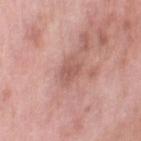Impression: Captured during whole-body skin photography for melanoma surveillance; the lesion was not biopsied. Acquisition and patient details: On the leg. This image is a 15 mm lesion crop taken from a total-body photograph. A female subject about 70 years old.